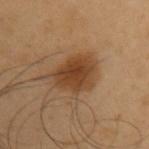Recorded during total-body skin imaging; not selected for excision or biopsy. Automated tile analysis of the lesion measured a lesion color around L≈40 a*≈19 b*≈32 in CIELAB, about 11 CIELAB-L* units darker than the surrounding skin, and a lesion-to-skin contrast of about 9 (normalized; higher = more distinct). It also reported a within-lesion color-variation index near 3.5/10. The lesion is on the arm. This is a cross-polarized tile. Cropped from a whole-body photographic skin survey; the tile spans about 15 mm. A male patient in their mid- to late 50s. The recorded lesion diameter is about 5.5 mm.